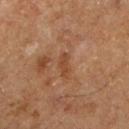biopsy status=total-body-photography surveillance lesion; no biopsy